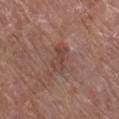Imaged during a routine full-body skin examination; the lesion was not biopsied and no histopathology is available. A female subject, aged 78–82. A roughly 15 mm field-of-view crop from a total-body skin photograph. On the right lower leg.